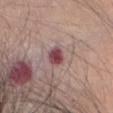No biopsy was performed on this lesion — it was imaged during a full skin examination and was not determined to be concerning. The lesion-visualizer software estimated a mean CIELAB color near L≈45 a*≈26 b*≈16, a lesion–skin lightness drop of about 15, and a normalized lesion–skin contrast near 11. The analysis additionally found internal color variation of about 5.5 on a 0–10 scale and radial color variation of about 2. It also reported lesion-presence confidence of about 100/100. A region of skin cropped from a whole-body photographic capture, roughly 15 mm wide. The lesion is on the head or neck. A female subject approximately 65 years of age. The recorded lesion diameter is about 2.5 mm. Captured under white-light illumination.